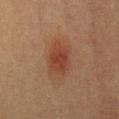Captured during whole-body skin photography for melanoma surveillance; the lesion was not biopsied. Automated image analysis of the tile measured a mean CIELAB color near L≈33 a*≈21 b*≈26 and a normalized border contrast of about 7. The software also gave a border-irregularity rating of about 2.5/10, a color-variation rating of about 3/10, and radial color variation of about 1. And it measured a classifier nevus-likeness of about 95/100 and a lesion-detection confidence of about 100/100. On the chest. A 15 mm close-up extracted from a 3D total-body photography capture. A male patient, aged around 40. The tile uses cross-polarized illumination.